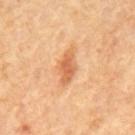follow-up: total-body-photography surveillance lesion; no biopsy | site: the mid back | image source: 15 mm crop, total-body photography | tile lighting: cross-polarized | diameter: about 4.5 mm | subject: male, aged approximately 65.